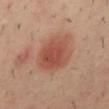workup = total-body-photography surveillance lesion; no biopsy | image-analysis metrics = a footprint of about 15 mm² and two-axis asymmetry of about 0.15; a peripheral color-asymmetry measure near 1 | illumination = cross-polarized illumination | body site = the mid back | image source = ~15 mm crop, total-body skin-cancer survey | lesion diameter = ~4.5 mm (longest diameter) | subject = male, aged around 40.